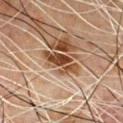<lesion>
<biopsy_status>not biopsied; imaged during a skin examination</biopsy_status>
<site>front of the torso</site>
<automated_metrics>
  <area_mm2_approx>6.0</area_mm2_approx>
  <eccentricity>0.95</eccentricity>
  <shape_asymmetry>0.35</shape_asymmetry>
  <cielab_L>34</cielab_L>
  <cielab_a>18</cielab_a>
  <cielab_b>27</cielab_b>
  <vs_skin_darker_L>13.0</vs_skin_darker_L>
  <vs_skin_contrast_norm>12.0</vs_skin_contrast_norm>
  <border_irregularity_0_10>5.0</border_irregularity_0_10>
  <color_variation_0_10>3.5</color_variation_0_10>
  <nevus_likeness_0_100>10</nevus_likeness_0_100>
  <lesion_detection_confidence_0_100>100</lesion_detection_confidence_0_100>
</automated_metrics>
<patient>
  <sex>male</sex>
  <age_approx>50</age_approx>
</patient>
<lesion_size>
  <long_diameter_mm_approx>4.5</long_diameter_mm_approx>
</lesion_size>
<image>
  <source>total-body photography crop</source>
  <field_of_view_mm>15</field_of_view_mm>
</image>
</lesion>